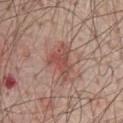Impression: Part of a total-body skin-imaging series; this lesion was reviewed on a skin check and was not flagged for biopsy. Clinical summary: Longest diameter approximately 5 mm. A 15 mm close-up extracted from a 3D total-body photography capture. A male patient aged 68 to 72. Imaged with white-light lighting. The lesion is located on the chest.